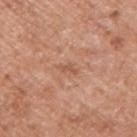Captured during whole-body skin photography for melanoma surveillance; the lesion was not biopsied. A 15 mm crop from a total-body photograph taken for skin-cancer surveillance. A male subject, aged 53 to 57. From the arm. Measured at roughly 2.5 mm in maximum diameter.